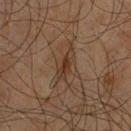  biopsy_status: not biopsied; imaged during a skin examination
  patient:
    sex: male
    age_approx: 60
  lighting: cross-polarized
  automated_metrics:
    border_irregularity_0_10: 4.5
    color_variation_0_10: 2.0
    peripheral_color_asymmetry: 0.5
    nevus_likeness_0_100: 5
  site: chest
  lesion_size:
    long_diameter_mm_approx: 4.0
  image:
    source: total-body photography crop
    field_of_view_mm: 15